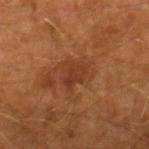Assessment: Captured during whole-body skin photography for melanoma surveillance; the lesion was not biopsied. Clinical summary: A 15 mm crop from a total-body photograph taken for skin-cancer surveillance. A male patient in their mid-60s. An algorithmic analysis of the crop reported a lesion area of about 6 mm², a shape eccentricity near 0.55, and a shape-asymmetry score of about 0.4 (0 = symmetric).